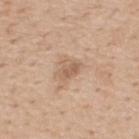Approximately 3 mm at its widest. Imaged with white-light lighting. On the upper back. The total-body-photography lesion software estimated an outline eccentricity of about 0.7 (0 = round, 1 = elongated) and two-axis asymmetry of about 0.35. The software also gave radial color variation of about 1. A roughly 15 mm field-of-view crop from a total-body skin photograph. The patient is a male about 60 years old.